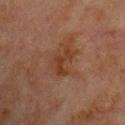Captured during whole-body skin photography for melanoma surveillance; the lesion was not biopsied.
About 3.5 mm across.
The subject is a male in their mid- to late 60s.
Captured under cross-polarized illumination.
Cropped from a whole-body photographic skin survey; the tile spans about 15 mm.
Located on the upper back.